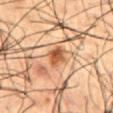Clinical impression:
Imaged during a routine full-body skin examination; the lesion was not biopsied and no histopathology is available.
Acquisition and patient details:
A male subject, aged 48 to 52. Automated image analysis of the tile measured a shape eccentricity near 0.8 and a symmetry-axis asymmetry near 0.25. It also reported an automated nevus-likeness rating near 100 out of 100 and a detector confidence of about 100 out of 100 that the crop contains a lesion. Longest diameter approximately 3.5 mm. Imaged with cross-polarized lighting. The lesion is located on the front of the torso. Cropped from a whole-body photographic skin survey; the tile spans about 15 mm.